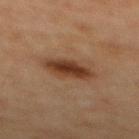The lesion was tiled from a total-body skin photograph and was not biopsied.